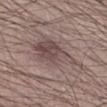biopsy status — no biopsy performed (imaged during a skin exam)
image source — ~15 mm tile from a whole-body skin photo
location — the leg
subject — male, aged approximately 30
image-analysis metrics — an area of roughly 20 mm² and two-axis asymmetry of about 0.45; a border-irregularity index near 5.5/10